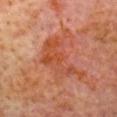workup = catalogued during a skin exam; not biopsied | location = the head or neck | patient = male, in their mid- to late 60s | imaging modality = ~15 mm crop, total-body skin-cancer survey | lighting = cross-polarized illumination.